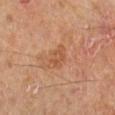Notes:
* workup — no biopsy performed (imaged during a skin exam)